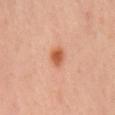The lesion was tiled from a total-body skin photograph and was not biopsied.
A male patient, about 65 years old.
A 15 mm close-up tile from a total-body photography series done for melanoma screening.
Approximately 2.5 mm at its widest.
Located on the mid back.
Captured under cross-polarized illumination.
Automated tile analysis of the lesion measured a lesion area of about 4 mm², an eccentricity of roughly 0.7, and a symmetry-axis asymmetry near 0.2. The analysis additionally found a lesion–skin lightness drop of about 12 and a normalized border contrast of about 9. And it measured a detector confidence of about 100 out of 100 that the crop contains a lesion.